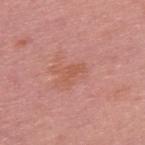Imaged during a routine full-body skin examination; the lesion was not biopsied and no histopathology is available. From the upper back. A male patient roughly 55 years of age. A lesion tile, about 15 mm wide, cut from a 3D total-body photograph. Automated tile analysis of the lesion measured a lesion color around L≈56 a*≈26 b*≈31 in CIELAB and roughly 6 lightness units darker than nearby skin. The analysis additionally found a classifier nevus-likeness of about 0/100 and a lesion-detection confidence of about 100/100.